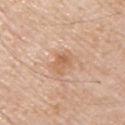Findings:
- biopsy status — imaged on a skin check; not biopsied
- body site — the left upper arm
- subject — male, aged approximately 60
- imaging modality — total-body-photography crop, ~15 mm field of view